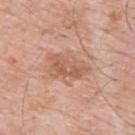Case summary:
– biopsy status · total-body-photography surveillance lesion; no biopsy
– anatomic site · the upper back
– lesion size · about 5.5 mm
– subject · male, aged approximately 60
– TBP lesion metrics · an area of roughly 13 mm² and a shape-asymmetry score of about 0.35 (0 = symmetric); a classifier nevus-likeness of about 0/100 and a lesion-detection confidence of about 100/100
– illumination · white-light illumination
– image source · ~15 mm crop, total-body skin-cancer survey